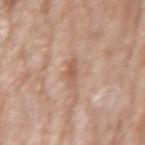<case>
<biopsy_status>not biopsied; imaged during a skin examination</biopsy_status>
<image>
  <source>total-body photography crop</source>
  <field_of_view_mm>15</field_of_view_mm>
</image>
<lesion_size>
  <long_diameter_mm_approx>3.5</long_diameter_mm_approx>
</lesion_size>
<patient>
  <sex>female</sex>
  <age_approx>75</age_approx>
</patient>
<lighting>white-light</lighting>
<automated_metrics>
  <cielab_L>58</cielab_L>
  <cielab_a>20</cielab_a>
  <cielab_b>29</cielab_b>
  <vs_skin_darker_L>9.0</vs_skin_darker_L>
  <border_irregularity_0_10>5.0</border_irregularity_0_10>
  <color_variation_0_10>0.5</color_variation_0_10>
  <peripheral_color_asymmetry>0.0</peripheral_color_asymmetry>
  <lesion_detection_confidence_0_100>100</lesion_detection_confidence_0_100>
</automated_metrics>
<site>left forearm</site>
</case>